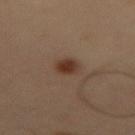Assessment:
This lesion was catalogued during total-body skin photography and was not selected for biopsy.
Clinical summary:
A male patient approximately 50 years of age. Located on the left thigh. Cropped from a total-body skin-imaging series; the visible field is about 15 mm. About 2.5 mm across. Automated image analysis of the tile measured a lesion area of about 5 mm², an eccentricity of roughly 0.45, and a symmetry-axis asymmetry near 0.15. And it measured a mean CIELAB color near L≈35 a*≈18 b*≈26 and roughly 10 lightness units darker than nearby skin. It also reported a border-irregularity index near 1/10 and peripheral color asymmetry of about 1. The analysis additionally found an automated nevus-likeness rating near 100 out of 100 and a detector confidence of about 100 out of 100 that the crop contains a lesion.